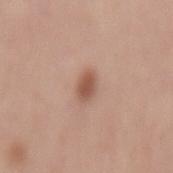{"biopsy_status": "not biopsied; imaged during a skin examination", "image": {"source": "total-body photography crop", "field_of_view_mm": 15}, "patient": {"sex": "male", "age_approx": 65}, "automated_metrics": {"border_irregularity_0_10": 1.5, "color_variation_0_10": 2.0, "peripheral_color_asymmetry": 0.5, "nevus_likeness_0_100": 95, "lesion_detection_confidence_0_100": 100}, "lighting": "white-light", "site": "mid back"}